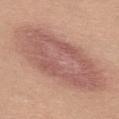Longest diameter approximately 12.5 mm.
This is a white-light tile.
This image is a 15 mm lesion crop taken from a total-body photograph.
Located on the left thigh.
A female subject in their mid- to late 40s.